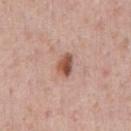- biopsy status — catalogued during a skin exam; not biopsied
- automated metrics — a lesion area of about 4.5 mm² and a shape eccentricity near 0.75; an automated nevus-likeness rating near 95 out of 100 and a lesion-detection confidence of about 100/100
- size — ≈3 mm
- acquisition — ~15 mm tile from a whole-body skin photo
- tile lighting — white-light illumination
- subject — male, aged approximately 40
- location — the chest